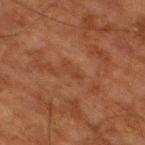This lesion was catalogued during total-body skin photography and was not selected for biopsy.
On the right thigh.
The recorded lesion diameter is about 2.5 mm.
A 15 mm close-up tile from a total-body photography series done for melanoma screening.
Captured under cross-polarized illumination.
An algorithmic analysis of the crop reported an area of roughly 2 mm², an eccentricity of roughly 0.95, and a symmetry-axis asymmetry near 0.45. And it measured an automated nevus-likeness rating near 0 out of 100 and a lesion-detection confidence of about 95/100.
The patient is a male approximately 80 years of age.